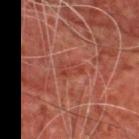Q: Was a biopsy performed?
A: total-body-photography surveillance lesion; no biopsy
Q: What are the patient's age and sex?
A: male, in their mid-60s
Q: What is the anatomic site?
A: the upper back
Q: How was this image acquired?
A: 15 mm crop, total-body photography
Q: Lesion size?
A: ~3 mm (longest diameter)
Q: Automated lesion metrics?
A: a footprint of about 5 mm², an eccentricity of roughly 0.85, and a symmetry-axis asymmetry near 0.3; border irregularity of about 3.5 on a 0–10 scale, a color-variation rating of about 2.5/10, and peripheral color asymmetry of about 1; a classifier nevus-likeness of about 0/100 and lesion-presence confidence of about 95/100
Q: How was the tile lit?
A: cross-polarized